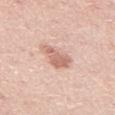follow-up: total-body-photography surveillance lesion; no biopsy | image-analysis metrics: a footprint of about 6.5 mm², a shape eccentricity near 0.85, and a shape-asymmetry score of about 0.35 (0 = symmetric); roughly 12 lightness units darker than nearby skin and a normalized lesion–skin contrast near 7; a border-irregularity index near 4/10, internal color variation of about 2 on a 0–10 scale, and peripheral color asymmetry of about 0.5; a nevus-likeness score of about 30/100 and a lesion-detection confidence of about 100/100 | body site: the front of the torso | lighting: white-light | subject: male, in their mid- to late 40s | image: 15 mm crop, total-body photography | lesion size: about 4 mm.